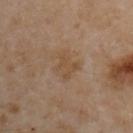The lesion was photographed on a routine skin check and not biopsied; there is no pathology result.
About 2.5 mm across.
On the right upper arm.
The total-body-photography lesion software estimated an area of roughly 5.5 mm², an eccentricity of roughly 0.5, and two-axis asymmetry of about 0.3. The software also gave a mean CIELAB color near L≈48 a*≈16 b*≈31, a lesion–skin lightness drop of about 6, and a normalized border contrast of about 5.5. The analysis additionally found a border-irregularity index near 4/10, a within-lesion color-variation index near 2/10, and peripheral color asymmetry of about 0.5.
Captured under cross-polarized illumination.
The patient is a male aged around 55.
Cropped from a whole-body photographic skin survey; the tile spans about 15 mm.